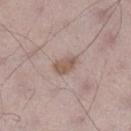Recorded during total-body skin imaging; not selected for excision or biopsy. Cropped from a whole-body photographic skin survey; the tile spans about 15 mm. The recorded lesion diameter is about 3 mm. A male subject, approximately 70 years of age. Located on the left lower leg.